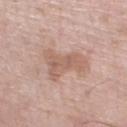Part of a total-body skin-imaging series; this lesion was reviewed on a skin check and was not flagged for biopsy.